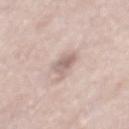Q: Is there a histopathology result?
A: no biopsy performed (imaged during a skin exam)
Q: Lesion location?
A: the mid back
Q: How large is the lesion?
A: ≈3.5 mm
Q: What lighting was used for the tile?
A: white-light illumination
Q: What is the imaging modality?
A: total-body-photography crop, ~15 mm field of view
Q: Patient demographics?
A: male, aged 73–77